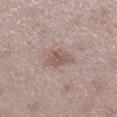Q: Lesion location?
A: the right lower leg
Q: Automated lesion metrics?
A: an automated nevus-likeness rating near 45 out of 100 and lesion-presence confidence of about 100/100
Q: What are the patient's age and sex?
A: female, aged 48–52
Q: How large is the lesion?
A: about 3.5 mm
Q: How was this image acquired?
A: ~15 mm tile from a whole-body skin photo
Q: Illumination type?
A: white-light illumination